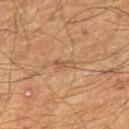| feature | finding |
|---|---|
| notes | catalogued during a skin exam; not biopsied |
| patient | male, aged 63–67 |
| site | the leg |
| imaging modality | ~15 mm crop, total-body skin-cancer survey |
| illumination | cross-polarized |
| size | ~2.5 mm (longest diameter) |
| image-analysis metrics | a lesion color around L≈44 a*≈16 b*≈29 in CIELAB and a normalized border contrast of about 5 |